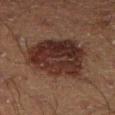Notes:
* follow-up · total-body-photography surveillance lesion; no biopsy
* lighting · cross-polarized
* image source · 15 mm crop, total-body photography
* location · the right lower leg
* size · ~6.5 mm (longest diameter)
* subject · male, roughly 45 years of age